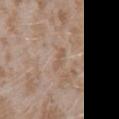notes: no biopsy performed (imaged during a skin exam) | image: ~15 mm crop, total-body skin-cancer survey | patient: female, aged 23 to 27 | location: the left forearm | diameter: ≈2.5 mm | illumination: white-light.